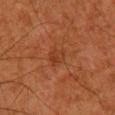{
  "biopsy_status": "not biopsied; imaged during a skin examination",
  "lighting": "cross-polarized",
  "site": "right lower leg",
  "patient": {
    "sex": "male",
    "age_approx": 80
  },
  "image": {
    "source": "total-body photography crop",
    "field_of_view_mm": 15
  }
}